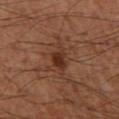This lesion was catalogued during total-body skin photography and was not selected for biopsy. This image is a 15 mm lesion crop taken from a total-body photograph. The lesion is on the left thigh. The patient is a male aged 53–57. Captured under cross-polarized illumination.